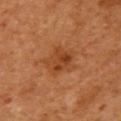The lesion was tiled from a total-body skin photograph and was not biopsied. The recorded lesion diameter is about 4.5 mm. Automated image analysis of the tile measured a normalized lesion–skin contrast near 6. And it measured border irregularity of about 3.5 on a 0–10 scale, internal color variation of about 5.5 on a 0–10 scale, and peripheral color asymmetry of about 2. This image is a 15 mm lesion crop taken from a total-body photograph. Imaged with cross-polarized lighting. A female patient aged approximately 55. From the right upper arm.